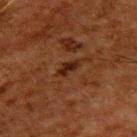Clinical impression: This lesion was catalogued during total-body skin photography and was not selected for biopsy. Clinical summary: The lesion-visualizer software estimated a footprint of about 3 mm², an outline eccentricity of about 0.9 (0 = round, 1 = elongated), and a symmetry-axis asymmetry near 0.4. Longest diameter approximately 3 mm. The lesion is located on the upper back. A roughly 15 mm field-of-view crop from a total-body skin photograph. This is a cross-polarized tile. The subject is a male aged approximately 60.